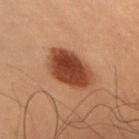Notes:
• biopsy status — no biopsy performed (imaged during a skin exam)
• lighting — cross-polarized
• patient — male, roughly 55 years of age
• TBP lesion metrics — an eccentricity of roughly 0.6 and two-axis asymmetry of about 0.15; a lesion color around L≈35 a*≈22 b*≈28 in CIELAB, roughly 14 lightness units darker than nearby skin, and a lesion-to-skin contrast of about 12 (normalized; higher = more distinct)
• anatomic site — the upper back
• diameter — about 5 mm
• imaging modality — 15 mm crop, total-body photography